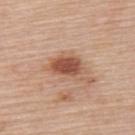• notes · no biopsy performed (imaged during a skin exam)
• lighting · white-light
• body site · the upper back
• diameter · ≈4 mm
• imaging modality · total-body-photography crop, ~15 mm field of view
• patient · female, aged approximately 65